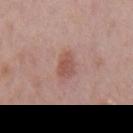Assessment:
Recorded during total-body skin imaging; not selected for excision or biopsy.
Acquisition and patient details:
Imaged with white-light lighting. Located on the mid back. Cropped from a total-body skin-imaging series; the visible field is about 15 mm. A male subject aged 73–77.